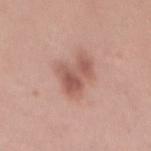Impression: Part of a total-body skin-imaging series; this lesion was reviewed on a skin check and was not flagged for biopsy. Image and clinical context: Cropped from a total-body skin-imaging series; the visible field is about 15 mm. On the mid back. A female patient aged around 25.